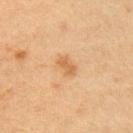Impression: The lesion was tiled from a total-body skin photograph and was not biopsied. Background: A roughly 15 mm field-of-view crop from a total-body skin photograph. A female subject aged around 45. Located on the right upper arm.